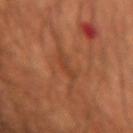Clinical impression: The lesion was photographed on a routine skin check and not biopsied; there is no pathology result. Context: Located on the left forearm. Automated tile analysis of the lesion measured an area of roughly 3 mm², an outline eccentricity of about 0.9 (0 = round, 1 = elongated), and two-axis asymmetry of about 0.45. The software also gave a mean CIELAB color near L≈41 a*≈25 b*≈34, about 6 CIELAB-L* units darker than the surrounding skin, and a lesion-to-skin contrast of about 5 (normalized; higher = more distinct). And it measured a color-variation rating of about 0/10 and a peripheral color-asymmetry measure near 0. A male patient, approximately 50 years of age. About 3 mm across. Captured under cross-polarized illumination. A roughly 15 mm field-of-view crop from a total-body skin photograph.